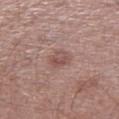Imaged during a routine full-body skin examination; the lesion was not biopsied and no histopathology is available. A male patient, aged 58 to 62. An algorithmic analysis of the crop reported a lesion area of about 5 mm², an eccentricity of roughly 0.7, and a symmetry-axis asymmetry near 0.2. It also reported a nevus-likeness score of about 10/100 and lesion-presence confidence of about 100/100. Captured under white-light illumination. Longest diameter approximately 3 mm. The lesion is located on the left lower leg. A 15 mm close-up tile from a total-body photography series done for melanoma screening.